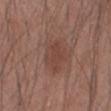Imaged during a routine full-body skin examination; the lesion was not biopsied and no histopathology is available.
The lesion is located on the left forearm.
The lesion's longest dimension is about 3.5 mm.
An algorithmic analysis of the crop reported border irregularity of about 4 on a 0–10 scale and radial color variation of about 0.5.
A male subject, about 45 years old.
A close-up tile cropped from a whole-body skin photograph, about 15 mm across.